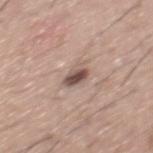Findings:
– biopsy status: no biopsy performed (imaged during a skin exam)
– lesion diameter: ~3 mm (longest diameter)
– lighting: white-light
– image source: ~15 mm tile from a whole-body skin photo
– patient: male, about 30 years old
– anatomic site: the mid back
– TBP lesion metrics: a mean CIELAB color near L≈52 a*≈16 b*≈22, about 14 CIELAB-L* units darker than the surrounding skin, and a lesion-to-skin contrast of about 9.5 (normalized; higher = more distinct)